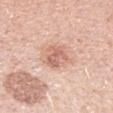Notes:
– follow-up — catalogued during a skin exam; not biopsied
– image source — ~15 mm tile from a whole-body skin photo
– subject — female, aged 28 to 32
– location — the right forearm
– lesion diameter — ~4 mm (longest diameter)
– automated metrics — a lesion–skin lightness drop of about 10; a border-irregularity rating of about 2/10, internal color variation of about 4.5 on a 0–10 scale, and a peripheral color-asymmetry measure near 1.5; a classifier nevus-likeness of about 20/100
– tile lighting — white-light